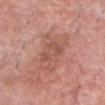The lesion was photographed on a routine skin check and not biopsied; there is no pathology result. This is a white-light tile. The lesion's longest dimension is about 6 mm. A roughly 15 mm field-of-view crop from a total-body skin photograph. The lesion is located on the head or neck. The lesion-visualizer software estimated an area of roughly 14 mm², a shape eccentricity near 0.85, and a shape-asymmetry score of about 0.3 (0 = symmetric). The software also gave a border-irregularity rating of about 4.5/10 and a peripheral color-asymmetry measure near 1. A male patient aged 58–62.